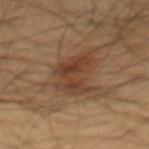No biopsy was performed on this lesion — it was imaged during a full skin examination and was not determined to be concerning. Automated image analysis of the tile measured an outline eccentricity of about 0.75 (0 = round, 1 = elongated) and a symmetry-axis asymmetry near 0.35. The analysis additionally found about 8 CIELAB-L* units darker than the surrounding skin and a normalized border contrast of about 7. The lesion's longest dimension is about 5 mm. The patient is a male aged approximately 60. The lesion is located on the abdomen. Captured under cross-polarized illumination. A close-up tile cropped from a whole-body skin photograph, about 15 mm across.